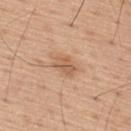The lesion was tiled from a total-body skin photograph and was not biopsied. Cropped from a whole-body photographic skin survey; the tile spans about 15 mm. Located on the upper back. A male subject, in their mid- to late 50s. Approximately 3 mm at its widest.